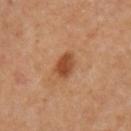Clinical impression: Imaged during a routine full-body skin examination; the lesion was not biopsied and no histopathology is available. Image and clinical context: The lesion is on the left upper arm. A female subject, aged 53 to 57. A roughly 15 mm field-of-view crop from a total-body skin photograph. Automated image analysis of the tile measured a lesion color around L≈44 a*≈23 b*≈34 in CIELAB, roughly 11 lightness units darker than nearby skin, and a normalized lesion–skin contrast near 8.5. The analysis additionally found border irregularity of about 1.5 on a 0–10 scale and a within-lesion color-variation index near 3/10. Longest diameter approximately 3 mm. Captured under cross-polarized illumination.